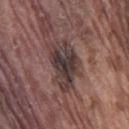No biopsy was performed on this lesion — it was imaged during a full skin examination and was not determined to be concerning.
On the leg.
This is a white-light tile.
Approximately 6 mm at its widest.
A 15 mm close-up tile from a total-body photography series done for melanoma screening.
The patient is a male approximately 80 years of age.